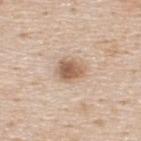Findings:
• notes · imaged on a skin check; not biopsied
• location · the upper back
• automated metrics · a border-irregularity index near 2.5/10, a color-variation rating of about 4.5/10, and radial color variation of about 1.5
• lighting · white-light
• image · 15 mm crop, total-body photography
• lesion size · about 3 mm
• subject · male, aged 43–47